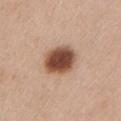The lesion was tiled from a total-body skin photograph and was not biopsied. A 15 mm close-up tile from a total-body photography series done for melanoma screening. Approximately 4.5 mm at its widest. The tile uses white-light illumination. Automated tile analysis of the lesion measured an outline eccentricity of about 0.55 (0 = round, 1 = elongated) and a symmetry-axis asymmetry near 0.15. The analysis additionally found a lesion color around L≈49 a*≈21 b*≈29 in CIELAB, about 19 CIELAB-L* units darker than the surrounding skin, and a normalized border contrast of about 13. The software also gave a border-irregularity index near 1/10, internal color variation of about 6 on a 0–10 scale, and peripheral color asymmetry of about 1.5. The analysis additionally found an automated nevus-likeness rating near 100 out of 100 and lesion-presence confidence of about 100/100. On the left upper arm. The subject is a male aged approximately 60.